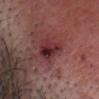| key | value |
|---|---|
| biopsy status | no biopsy performed (imaged during a skin exam) |
| size | about 3.5 mm |
| lighting | white-light |
| image source | total-body-photography crop, ~15 mm field of view |
| anatomic site | the head or neck |
| patient | male, aged around 55 |
| image-analysis metrics | two-axis asymmetry of about 0.3; an average lesion color of about L≈31 a*≈27 b*≈17 (CIELAB) and about 10 CIELAB-L* units darker than the surrounding skin; a border-irregularity rating of about 3/10, internal color variation of about 9 on a 0–10 scale, and peripheral color asymmetry of about 3; a nevus-likeness score of about 0/100 |